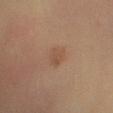Part of a total-body skin-imaging series; this lesion was reviewed on a skin check and was not flagged for biopsy. The tile uses cross-polarized illumination. The lesion is located on the leg. Automated tile analysis of the lesion measured an area of roughly 4 mm², a shape eccentricity near 0.7, and a symmetry-axis asymmetry near 0.25. A 15 mm crop from a total-body photograph taken for skin-cancer surveillance. A female patient about 65 years old. About 2.5 mm across.